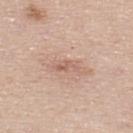No biopsy was performed on this lesion — it was imaged during a full skin examination and was not determined to be concerning.
A lesion tile, about 15 mm wide, cut from a 3D total-body photograph.
A male patient, about 45 years old.
From the back.